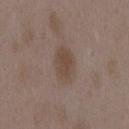Findings:
• biopsy status: catalogued during a skin exam; not biopsied
• subject: female, in their mid- to late 30s
• image-analysis metrics: a shape eccentricity near 0.75; an average lesion color of about L≈45 a*≈14 b*≈24 (CIELAB), roughly 7 lightness units darker than nearby skin, and a normalized border contrast of about 6.5
• image source: ~15 mm tile from a whole-body skin photo
• tile lighting: white-light illumination
• lesion size: about 4 mm
• location: the mid back